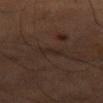Impression:
The lesion was tiled from a total-body skin photograph and was not biopsied.
Acquisition and patient details:
The total-body-photography lesion software estimated a mean CIELAB color near L≈23 a*≈12 b*≈19 and roughly 4 lightness units darker than nearby skin. The software also gave a border-irregularity index near 5.5/10, internal color variation of about 0 on a 0–10 scale, and peripheral color asymmetry of about 0. About 3 mm across. From the leg. A 15 mm close-up extracted from a 3D total-body photography capture. Imaged with cross-polarized lighting. The subject is a male aged around 55.